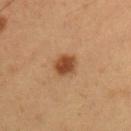biopsy_status: not biopsied; imaged during a skin examination
site: front of the torso
automated_metrics:
  cielab_L: 46
  cielab_a: 23
  cielab_b: 35
  vs_skin_darker_L: 13.0
  vs_skin_contrast_norm: 9.5
  nevus_likeness_0_100: 100
  lesion_detection_confidence_0_100: 100
patient:
  sex: male
  age_approx: 55
image:
  source: total-body photography crop
  field_of_view_mm: 15
lesion_size:
  long_diameter_mm_approx: 2.5